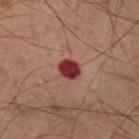<case>
<biopsy_status>not biopsied; imaged during a skin examination</biopsy_status>
<image>
  <source>total-body photography crop</source>
  <field_of_view_mm>15</field_of_view_mm>
</image>
<patient>
  <sex>male</sex>
  <age_approx>60</age_approx>
</patient>
<site>right lower leg</site>
</case>